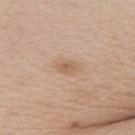notes = no biopsy performed (imaged during a skin exam) | diameter = about 2.5 mm | lighting = white-light illumination | site = the chest | image-analysis metrics = a lesion area of about 3.5 mm², an eccentricity of roughly 0.75, and a symmetry-axis asymmetry near 0.2; a lesion color around L≈60 a*≈18 b*≈32 in CIELAB; a border-irregularity rating of about 2/10 and a within-lesion color-variation index near 3/10 | patient = male, in their mid- to late 50s | acquisition = ~15 mm crop, total-body skin-cancer survey.